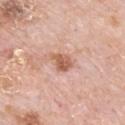A lesion tile, about 15 mm wide, cut from a 3D total-body photograph. This is a white-light tile. The recorded lesion diameter is about 2.5 mm. Located on the back. The total-body-photography lesion software estimated an area of roughly 4.5 mm², an outline eccentricity of about 0.65 (0 = round, 1 = elongated), and a shape-asymmetry score of about 0.35 (0 = symmetric). The analysis additionally found a lesion color around L≈59 a*≈24 b*≈32 in CIELAB and roughly 12 lightness units darker than nearby skin. The software also gave a classifier nevus-likeness of about 45/100. A male patient, aged 78–82.